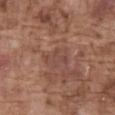subject: male, in their mid-70s
imaging modality: total-body-photography crop, ~15 mm field of view
site: the abdomen